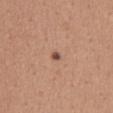Captured during whole-body skin photography for melanoma surveillance; the lesion was not biopsied. A region of skin cropped from a whole-body photographic capture, roughly 15 mm wide. A female subject about 45 years old. On the chest.